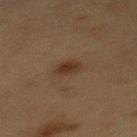Findings:
• notes — total-body-photography surveillance lesion; no biopsy
• acquisition — ~15 mm crop, total-body skin-cancer survey
• lesion diameter — about 2.5 mm
• TBP lesion metrics — an area of roughly 3.5 mm², an eccentricity of roughly 0.7, and a symmetry-axis asymmetry near 0.25; an average lesion color of about L≈28 a*≈15 b*≈24 (CIELAB), a lesion–skin lightness drop of about 8, and a normalized lesion–skin contrast near 8; a within-lesion color-variation index near 2.5/10
• patient — female, aged 38 to 42
• body site — the mid back
• tile lighting — cross-polarized